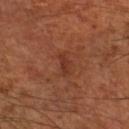Assessment:
This lesion was catalogued during total-body skin photography and was not selected for biopsy.
Background:
The lesion's longest dimension is about 3 mm. Located on the right thigh. A 15 mm close-up extracted from a 3D total-body photography capture. Automated image analysis of the tile measured a border-irregularity rating of about 3/10, internal color variation of about 0 on a 0–10 scale, and peripheral color asymmetry of about 0. This is a cross-polarized tile. The subject is a male aged 68–72.